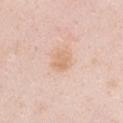About 2.5 mm across. Imaged with white-light lighting. Automated tile analysis of the lesion measured a mean CIELAB color near L≈70 a*≈19 b*≈33 and a normalized lesion–skin contrast near 6. The analysis additionally found a border-irregularity index near 2/10 and a color-variation rating of about 2.5/10. From the front of the torso. A female subject roughly 30 years of age. A 15 mm crop from a total-body photograph taken for skin-cancer surveillance.